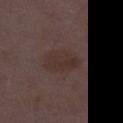Assessment:
The lesion was photographed on a routine skin check and not biopsied; there is no pathology result.
Context:
Imaged with white-light lighting. Located on the leg. An algorithmic analysis of the crop reported a footprint of about 11 mm², an eccentricity of roughly 0.8, and two-axis asymmetry of about 0.15. The analysis additionally found a nevus-likeness score of about 0/100. A 15 mm close-up extracted from a 3D total-body photography capture. A female patient aged approximately 30.